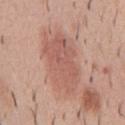Clinical impression: No biopsy was performed on this lesion — it was imaged during a full skin examination and was not determined to be concerning. Clinical summary: About 7.5 mm across. A roughly 15 mm field-of-view crop from a total-body skin photograph. The total-body-photography lesion software estimated an area of roughly 23 mm², a shape eccentricity near 0.85, and a shape-asymmetry score of about 0.25 (0 = symmetric). The analysis additionally found a mean CIELAB color near L≈57 a*≈23 b*≈27, about 9 CIELAB-L* units darker than the surrounding skin, and a lesion-to-skin contrast of about 6 (normalized; higher = more distinct). The analysis additionally found a border-irregularity rating of about 3/10, internal color variation of about 3.5 on a 0–10 scale, and radial color variation of about 1. The lesion is on the chest. The tile uses white-light illumination. The subject is a male in their 40s.